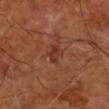workup = catalogued during a skin exam; not biopsied, automated lesion analysis = an area of roughly 3 mm² and a shape eccentricity near 0.9; a detector confidence of about 100 out of 100 that the crop contains a lesion, imaging modality = ~15 mm tile from a whole-body skin photo, tile lighting = cross-polarized illumination, site = the left forearm, patient = aged 63 to 67, lesion size = about 2.5 mm.